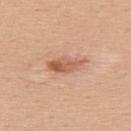Imaged during a routine full-body skin examination; the lesion was not biopsied and no histopathology is available.
The subject is a female aged 43 to 47.
Measured at roughly 4.5 mm in maximum diameter.
The tile uses white-light illumination.
Located on the upper back.
Automated tile analysis of the lesion measured a footprint of about 6 mm², an eccentricity of roughly 0.9, and two-axis asymmetry of about 0.3. It also reported an average lesion color of about L≈59 a*≈24 b*≈33 (CIELAB), about 12 CIELAB-L* units darker than the surrounding skin, and a normalized border contrast of about 7.5. The analysis additionally found an automated nevus-likeness rating near 70 out of 100.
Cropped from a total-body skin-imaging series; the visible field is about 15 mm.